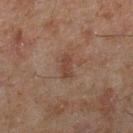Acquisition and patient details:
The recorded lesion diameter is about 3 mm. A male subject about 45 years old. Cropped from a total-body skin-imaging series; the visible field is about 15 mm. On the right lower leg. Captured under cross-polarized illumination.